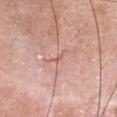<record>
<biopsy_status>not biopsied; imaged during a skin examination</biopsy_status>
<patient>
  <sex>female</sex>
  <age_approx>60</age_approx>
</patient>
<lesion_size>
  <long_diameter_mm_approx>1.0</long_diameter_mm_approx>
</lesion_size>
<automated_metrics>
  <cielab_L>59</cielab_L>
  <cielab_a>26</cielab_a>
  <cielab_b>25</cielab_b>
  <vs_skin_darker_L>8.0</vs_skin_darker_L>
  <border_irregularity_0_10>2.0</border_irregularity_0_10>
  <peripheral_color_asymmetry>0.0</peripheral_color_asymmetry>
  <nevus_likeness_0_100>0</nevus_likeness_0_100>
  <lesion_detection_confidence_0_100>5</lesion_detection_confidence_0_100>
</automated_metrics>
<lighting>white-light</lighting>
<image>
  <source>total-body photography crop</source>
  <field_of_view_mm>15</field_of_view_mm>
</image>
<site>head or neck</site>
</record>